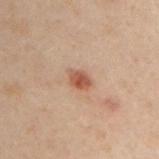Part of a total-body skin-imaging series; this lesion was reviewed on a skin check and was not flagged for biopsy.
About 2.5 mm across.
The lesion is located on the left upper arm.
A male subject, approximately 50 years of age.
A lesion tile, about 15 mm wide, cut from a 3D total-body photograph.
The tile uses cross-polarized illumination.